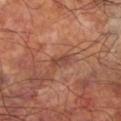No biopsy was performed on this lesion — it was imaged during a full skin examination and was not determined to be concerning. A close-up tile cropped from a whole-body skin photograph, about 15 mm across. Captured under cross-polarized illumination. The lesion is on the leg. The recorded lesion diameter is about 2.5 mm. A male patient, approximately 60 years of age.